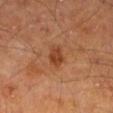anatomic site = the left lower leg
subject = male, aged 63–67
image = ~15 mm crop, total-body skin-cancer survey
illumination = cross-polarized illumination
lesion diameter = about 3 mm